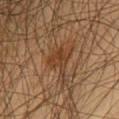Impression: Part of a total-body skin-imaging series; this lesion was reviewed on a skin check and was not flagged for biopsy. Clinical summary: A close-up tile cropped from a whole-body skin photograph, about 15 mm across. A male patient, approximately 60 years of age. The lesion is located on the chest. An algorithmic analysis of the crop reported a lesion area of about 7.5 mm² and an outline eccentricity of about 0.7 (0 = round, 1 = elongated). It also reported about 7 CIELAB-L* units darker than the surrounding skin and a lesion-to-skin contrast of about 7 (normalized; higher = more distinct). The software also gave an automated nevus-likeness rating near 20 out of 100. Measured at roughly 4 mm in maximum diameter.